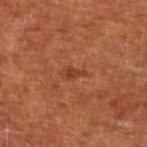Q: Was a biopsy performed?
A: total-body-photography surveillance lesion; no biopsy
Q: Who is the patient?
A: roughly 65 years of age
Q: What lighting was used for the tile?
A: cross-polarized illumination
Q: Lesion size?
A: ~2.5 mm (longest diameter)
Q: How was this image acquired?
A: 15 mm crop, total-body photography
Q: What is the anatomic site?
A: the leg